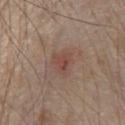Recorded during total-body skin imaging; not selected for excision or biopsy. Located on the front of the torso. A roughly 15 mm field-of-view crop from a total-body skin photograph. A male patient aged around 80. About 3 mm across.